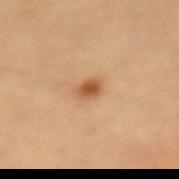Q: Lesion size?
A: about 2.5 mm
Q: Where on the body is the lesion?
A: the mid back
Q: Who is the patient?
A: female, aged 63–67
Q: How was the tile lit?
A: cross-polarized
Q: What kind of image is this?
A: total-body-photography crop, ~15 mm field of view
Q: What did automated image analysis measure?
A: about 10 CIELAB-L* units darker than the surrounding skin and a normalized border contrast of about 8